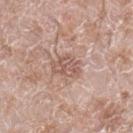Impression: The lesion was photographed on a routine skin check and not biopsied; there is no pathology result. Image and clinical context: A male patient aged approximately 60. From the right lower leg. The recorded lesion diameter is about 4 mm. This is a white-light tile. A lesion tile, about 15 mm wide, cut from a 3D total-body photograph. The lesion-visualizer software estimated a normalized lesion–skin contrast near 6. It also reported a border-irregularity index near 3.5/10 and a peripheral color-asymmetry measure near 1.5. The analysis additionally found an automated nevus-likeness rating near 0 out of 100 and lesion-presence confidence of about 100/100.